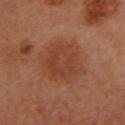Captured during whole-body skin photography for melanoma surveillance; the lesion was not biopsied.
On the head or neck.
A female patient aged approximately 60.
This image is a 15 mm lesion crop taken from a total-body photograph.
The recorded lesion diameter is about 5.5 mm.
Captured under cross-polarized illumination.